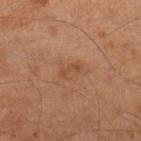Q: Was this lesion biopsied?
A: imaged on a skin check; not biopsied
Q: How was this image acquired?
A: 15 mm crop, total-body photography
Q: Illumination type?
A: cross-polarized illumination
Q: What is the anatomic site?
A: the right lower leg
Q: Who is the patient?
A: male, aged 63–67
Q: Lesion size?
A: ~2.5 mm (longest diameter)
Q: What did automated image analysis measure?
A: a footprint of about 3 mm², an outline eccentricity of about 0.85 (0 = round, 1 = elongated), and a symmetry-axis asymmetry near 0.35; a lesion color around L≈46 a*≈22 b*≈32 in CIELAB and a normalized border contrast of about 5; border irregularity of about 4 on a 0–10 scale, internal color variation of about 0 on a 0–10 scale, and a peripheral color-asymmetry measure near 0; a classifier nevus-likeness of about 0/100 and a lesion-detection confidence of about 100/100